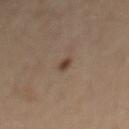<record>
<biopsy_status>not biopsied; imaged during a skin examination</biopsy_status>
<image>
  <source>total-body photography crop</source>
  <field_of_view_mm>15</field_of_view_mm>
</image>
<site>mid back</site>
<lighting>cross-polarized</lighting>
<patient>
  <sex>male</sex>
  <age_approx>55</age_approx>
</patient>
</record>